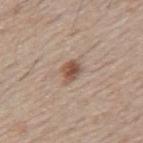Q: Is there a histopathology result?
A: no biopsy performed (imaged during a skin exam)
Q: Automated lesion metrics?
A: an outline eccentricity of about 0.65 (0 = round, 1 = elongated) and a shape-asymmetry score of about 0.3 (0 = symmetric); an average lesion color of about L≈52 a*≈19 b*≈27 (CIELAB), roughly 13 lightness units darker than nearby skin, and a normalized lesion–skin contrast near 9; a border-irregularity rating of about 3/10 and internal color variation of about 4.5 on a 0–10 scale
Q: Lesion size?
A: ≈3 mm
Q: Patient demographics?
A: male, aged 53 to 57
Q: Where on the body is the lesion?
A: the mid back
Q: How was this image acquired?
A: 15 mm crop, total-body photography
Q: Illumination type?
A: white-light